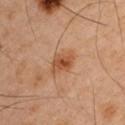Recorded during total-body skin imaging; not selected for excision or biopsy. An algorithmic analysis of the crop reported a border-irregularity index near 2.5/10, a color-variation rating of about 4.5/10, and peripheral color asymmetry of about 1. The analysis additionally found an automated nevus-likeness rating near 80 out of 100. Located on the left upper arm. A male patient, aged around 45. A roughly 15 mm field-of-view crop from a total-body skin photograph. Captured under cross-polarized illumination. Measured at roughly 3.5 mm in maximum diameter.